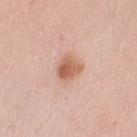biopsy status = total-body-photography surveillance lesion; no biopsy | anatomic site = the left upper arm | acquisition = total-body-photography crop, ~15 mm field of view | size = ≈3 mm | subject = female, in their 40s | illumination = white-light | automated metrics = an average lesion color of about L≈62 a*≈23 b*≈31 (CIELAB), a lesion–skin lightness drop of about 12, and a normalized border contrast of about 8; a border-irregularity rating of about 2/10 and a peripheral color-asymmetry measure near 2; an automated nevus-likeness rating near 95 out of 100 and a detector confidence of about 100 out of 100 that the crop contains a lesion.